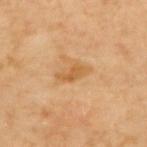Imaged during a routine full-body skin examination; the lesion was not biopsied and no histopathology is available. The subject is a female aged 63–67. The lesion is located on the upper back. A roughly 15 mm field-of-view crop from a total-body skin photograph. Longest diameter approximately 3.5 mm.